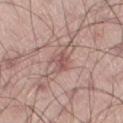Findings:
* lighting · white-light illumination
* image · 15 mm crop, total-body photography
* subject · male, aged 43–47
* lesion size · about 2.5 mm
* site · the right thigh